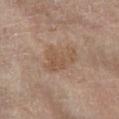Q: Was a biopsy performed?
A: total-body-photography surveillance lesion; no biopsy
Q: What lighting was used for the tile?
A: cross-polarized
Q: Where on the body is the lesion?
A: the left lower leg
Q: What is the imaging modality?
A: ~15 mm crop, total-body skin-cancer survey
Q: Patient demographics?
A: female, approximately 75 years of age